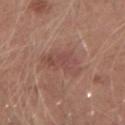The lesion was photographed on a routine skin check and not biopsied; there is no pathology result.
A male subject, about 25 years old.
The recorded lesion diameter is about 4 mm.
On the left forearm.
This image is a 15 mm lesion crop taken from a total-body photograph.